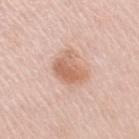Impression: No biopsy was performed on this lesion — it was imaged during a full skin examination and was not determined to be concerning. Acquisition and patient details: Cropped from a whole-body photographic skin survey; the tile spans about 15 mm. This is a white-light tile. Located on the right upper arm. An algorithmic analysis of the crop reported a border-irregularity index near 3.5/10, a within-lesion color-variation index near 4.5/10, and a peripheral color-asymmetry measure near 1.5. A female subject, in their mid-60s.